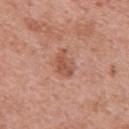follow-up=imaged on a skin check; not biopsied
TBP lesion metrics=a footprint of about 5 mm² and a shape eccentricity near 0.8; a border-irregularity index near 2.5/10 and radial color variation of about 0.5
acquisition=~15 mm crop, total-body skin-cancer survey
lesion diameter=about 3 mm
lighting=white-light
site=the upper back
patient=female, about 50 years old